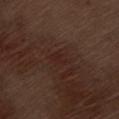follow-up: total-body-photography surveillance lesion; no biopsy | image: total-body-photography crop, ~15 mm field of view | site: the left thigh | illumination: white-light illumination | TBP lesion metrics: a footprint of about 3.5 mm², an eccentricity of roughly 0.85, and a symmetry-axis asymmetry near 0.2; an average lesion color of about L≈23 a*≈19 b*≈21 (CIELAB), a lesion–skin lightness drop of about 4, and a normalized lesion–skin contrast near 4.5; a color-variation rating of about 1.5/10 and radial color variation of about 0.5; a detector confidence of about 100 out of 100 that the crop contains a lesion | diameter: about 3 mm | subject: male, roughly 70 years of age.